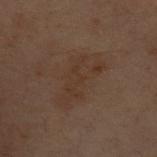| key | value |
|---|---|
| workup | total-body-photography surveillance lesion; no biopsy |
| image | total-body-photography crop, ~15 mm field of view |
| anatomic site | the left forearm |
| subject | female, aged around 55 |